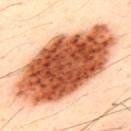workup: no biopsy performed (imaged during a skin exam); lighting: cross-polarized; body site: the upper back; subject: male, aged approximately 50; image: ~15 mm tile from a whole-body skin photo; lesion size: ≈13.5 mm.